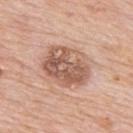Assessment: The lesion was photographed on a routine skin check and not biopsied; there is no pathology result. Context: The patient is a male about 80 years old. The lesion is located on the upper back. A 15 mm crop from a total-body photograph taken for skin-cancer surveillance.